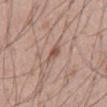Q: Is there a histopathology result?
A: no biopsy performed (imaged during a skin exam)
Q: Where on the body is the lesion?
A: the abdomen
Q: Illumination type?
A: white-light illumination
Q: Patient demographics?
A: male, aged around 55
Q: How was this image acquired?
A: total-body-photography crop, ~15 mm field of view
Q: What is the lesion's diameter?
A: ~2.5 mm (longest diameter)
Q: What did automated image analysis measure?
A: a border-irregularity index near 3/10, internal color variation of about 1.5 on a 0–10 scale, and radial color variation of about 0.5; an automated nevus-likeness rating near 20 out of 100 and a detector confidence of about 100 out of 100 that the crop contains a lesion